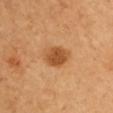{"biopsy_status": "not biopsied; imaged during a skin examination", "site": "left upper arm", "patient": {"sex": "female", "age_approx": 55}, "lesion_size": {"long_diameter_mm_approx": 3.5}, "lighting": "cross-polarized", "automated_metrics": {"area_mm2_approx": 8.5, "eccentricity": 0.55, "shape_asymmetry": 0.15, "cielab_L": 54, "cielab_a": 25, "cielab_b": 43}, "image": {"source": "total-body photography crop", "field_of_view_mm": 15}}